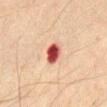notes = total-body-photography surveillance lesion; no biopsy
image = ~15 mm tile from a whole-body skin photo
subject = male, aged 68–72
location = the lower back
lesion size = ≈3 mm
lighting = cross-polarized